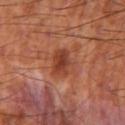| key | value |
|---|---|
| follow-up | imaged on a skin check; not biopsied |
| site | the right thigh |
| automated metrics | a footprint of about 8 mm², a shape eccentricity near 0.75, and two-axis asymmetry of about 0.2; an average lesion color of about L≈42 a*≈28 b*≈33 (CIELAB), roughly 10 lightness units darker than nearby skin, and a normalized border contrast of about 7.5; border irregularity of about 1.5 on a 0–10 scale and a peripheral color-asymmetry measure near 1.5; a classifier nevus-likeness of about 5/100 and a lesion-detection confidence of about 100/100 |
| size | ≈3.5 mm |
| lighting | cross-polarized |
| subject | male, about 55 years old |
| image | total-body-photography crop, ~15 mm field of view |